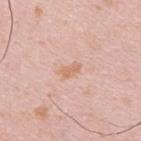The patient is a male approximately 40 years of age.
The recorded lesion diameter is about 2.5 mm.
An algorithmic analysis of the crop reported an area of roughly 3 mm². The software also gave a lesion color around L≈67 a*≈21 b*≈32 in CIELAB, about 7 CIELAB-L* units darker than the surrounding skin, and a normalized lesion–skin contrast near 6.5. It also reported a border-irregularity index near 3.5/10. It also reported a nevus-likeness score of about 0/100 and a lesion-detection confidence of about 100/100.
Captured under white-light illumination.
A 15 mm crop from a total-body photograph taken for skin-cancer surveillance.
On the upper back.